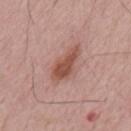{
  "biopsy_status": "not biopsied; imaged during a skin examination",
  "patient": {
    "sex": "male",
    "age_approx": 55
  },
  "lighting": "white-light",
  "image": {
    "source": "total-body photography crop",
    "field_of_view_mm": 15
  },
  "lesion_size": {
    "long_diameter_mm_approx": 5.5
  }
}